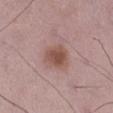Cropped from a total-body skin-imaging series; the visible field is about 15 mm.
From the right lower leg.
A male patient, aged 48–52.
Longest diameter approximately 3.5 mm.